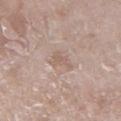Recorded during total-body skin imaging; not selected for excision or biopsy. The lesion is on the leg. Longest diameter approximately 3 mm. A female subject aged 73 to 77. A region of skin cropped from a whole-body photographic capture, roughly 15 mm wide.